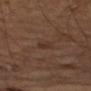biopsy_status: not biopsied; imaged during a skin examination
image:
  source: total-body photography crop
  field_of_view_mm: 15
site: left forearm
lesion_size:
  long_diameter_mm_approx: 2.5
lighting: cross-polarized
patient:
  sex: male
  age_approx: 65